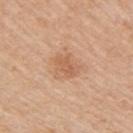Q: Was a biopsy performed?
A: catalogued during a skin exam; not biopsied
Q: What is the imaging modality?
A: ~15 mm tile from a whole-body skin photo
Q: What did automated image analysis measure?
A: an area of roughly 4.5 mm², a shape eccentricity near 0.7, and a symmetry-axis asymmetry near 0.35; a classifier nevus-likeness of about 30/100 and lesion-presence confidence of about 100/100
Q: What lighting was used for the tile?
A: white-light illumination
Q: Who is the patient?
A: female, in their 50s
Q: Lesion location?
A: the right upper arm